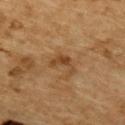| key | value |
|---|---|
| biopsy status | imaged on a skin check; not biopsied |
| patient | male, aged approximately 85 or older |
| imaging modality | ~15 mm crop, total-body skin-cancer survey |
| diameter | about 3.5 mm |
| site | the mid back |